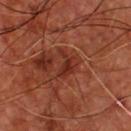<case>
<biopsy_status>not biopsied; imaged during a skin examination</biopsy_status>
<patient>
  <sex>male</sex>
  <age_approx>65</age_approx>
</patient>
<lesion_size>
  <long_diameter_mm_approx>3.0</long_diameter_mm_approx>
</lesion_size>
<image>
  <source>total-body photography crop</source>
  <field_of_view_mm>15</field_of_view_mm>
</image>
<lighting>cross-polarized</lighting>
<site>chest</site>
</case>